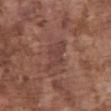| feature | finding |
|---|---|
| workup | catalogued during a skin exam; not biopsied |
| lesion size | about 4 mm |
| lighting | white-light illumination |
| subject | male, aged around 75 |
| location | the abdomen |
| automated metrics | a footprint of about 7.5 mm² and a shape eccentricity near 0.8; an average lesion color of about L≈41 a*≈21 b*≈23 (CIELAB) and a lesion-to-skin contrast of about 5.5 (normalized; higher = more distinct); a lesion-detection confidence of about 95/100 |
| acquisition | 15 mm crop, total-body photography |